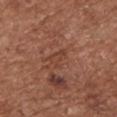Impression:
This lesion was catalogued during total-body skin photography and was not selected for biopsy.
Background:
Longest diameter approximately 2.5 mm. Imaged with white-light lighting. The lesion-visualizer software estimated a mean CIELAB color near L≈42 a*≈24 b*≈29, roughly 5 lightness units darker than nearby skin, and a normalized lesion–skin contrast near 4.5. It also reported radial color variation of about 0.5. This image is a 15 mm lesion crop taken from a total-body photograph. A female patient roughly 75 years of age. The lesion is on the chest.